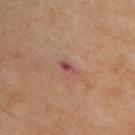biopsy_status: not biopsied; imaged during a skin examination
site: upper back
image:
  source: total-body photography crop
  field_of_view_mm: 15
patient:
  sex: male
  age_approx: 70